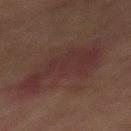{
  "biopsy_status": "not biopsied; imaged during a skin examination",
  "image": {
    "source": "total-body photography crop",
    "field_of_view_mm": 15
  },
  "patient": {
    "sex": "male",
    "age_approx": 65
  },
  "site": "front of the torso"
}